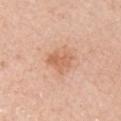notes=no biopsy performed (imaged during a skin exam).